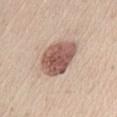Imaged during a routine full-body skin examination; the lesion was not biopsied and no histopathology is available.
On the abdomen.
A region of skin cropped from a whole-body photographic capture, roughly 15 mm wide.
The tile uses white-light illumination.
A female subject, aged around 30.